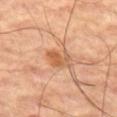Captured during whole-body skin photography for melanoma surveillance; the lesion was not biopsied.
A close-up tile cropped from a whole-body skin photograph, about 15 mm across.
From the left thigh.
Approximately 3 mm at its widest.
A male patient, aged approximately 65.
This is a cross-polarized tile.